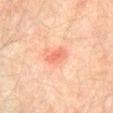workup: total-body-photography surveillance lesion; no biopsy
lesion size: ≈2.5 mm
location: the abdomen
lighting: cross-polarized
imaging modality: 15 mm crop, total-body photography
subject: male, approximately 75 years of age